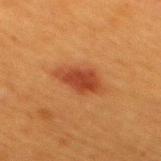<lesion>
  <biopsy_status>not biopsied; imaged during a skin examination</biopsy_status>
  <image>
    <source>total-body photography crop</source>
    <field_of_view_mm>15</field_of_view_mm>
  </image>
  <lighting>cross-polarized</lighting>
  <automated_metrics>
    <area_mm2_approx>9.5</area_mm2_approx>
    <eccentricity>0.8</eccentricity>
    <shape_asymmetry>0.2</shape_asymmetry>
  </automated_metrics>
  <patient>
    <sex>female</sex>
    <age_approx>40</age_approx>
  </patient>
  <site>back</site>
</lesion>